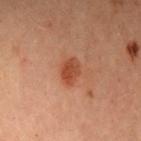Background:
Cropped from a whole-body photographic skin survey; the tile spans about 15 mm. The lesion's longest dimension is about 3 mm. Imaged with cross-polarized lighting. The subject is a female aged 28–32. The lesion is located on the head or neck.